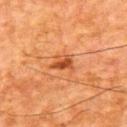Captured during whole-body skin photography for melanoma surveillance; the lesion was not biopsied. A 15 mm close-up extracted from a 3D total-body photography capture. The subject is a male aged 63–67. This is a cross-polarized tile. About 3 mm across. The lesion-visualizer software estimated an eccentricity of roughly 0.75. And it measured a border-irregularity index near 4/10, internal color variation of about 4.5 on a 0–10 scale, and radial color variation of about 1.5. The lesion is on the upper back.